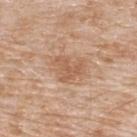anatomic site: the upper back
automated lesion analysis: a lesion area of about 7 mm²; a mean CIELAB color near L≈58 a*≈21 b*≈33, about 8 CIELAB-L* units darker than the surrounding skin, and a lesion-to-skin contrast of about 6 (normalized; higher = more distinct); a within-lesion color-variation index near 2/10 and radial color variation of about 0.5
image source: ~15 mm tile from a whole-body skin photo
illumination: white-light illumination
diameter: about 3.5 mm
subject: male, aged around 80